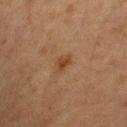Q: Is there a histopathology result?
A: catalogued during a skin exam; not biopsied
Q: What is the imaging modality?
A: 15 mm crop, total-body photography
Q: How large is the lesion?
A: ~2.5 mm (longest diameter)
Q: Automated lesion metrics?
A: an area of roughly 3 mm² and a symmetry-axis asymmetry near 0.3; an automated nevus-likeness rating near 70 out of 100 and a detector confidence of about 100 out of 100 that the crop contains a lesion
Q: Who is the patient?
A: male, in their mid-60s
Q: What is the anatomic site?
A: the arm
Q: What lighting was used for the tile?
A: cross-polarized illumination